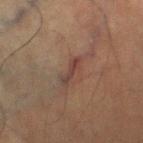follow-up = catalogued during a skin exam; not biopsied
lesion size = ~3 mm (longest diameter)
lighting = cross-polarized illumination
site = the right thigh
acquisition = 15 mm crop, total-body photography
patient = male, approximately 60 years of age
TBP lesion metrics = a border-irregularity index near 6/10, a within-lesion color-variation index near 0/10, and peripheral color asymmetry of about 0; a classifier nevus-likeness of about 0/100 and a lesion-detection confidence of about 95/100